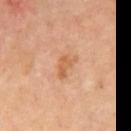follow-up — total-body-photography surveillance lesion; no biopsy
tile lighting — cross-polarized
lesion size — about 3.5 mm
subject — male, approximately 70 years of age
imaging modality — 15 mm crop, total-body photography
site — the chest